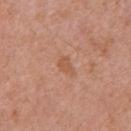The lesion was tiled from a total-body skin photograph and was not biopsied. Located on the chest. Imaged with white-light lighting. Longest diameter approximately 2.5 mm. A male patient about 70 years old. A 15 mm crop from a total-body photograph taken for skin-cancer surveillance.